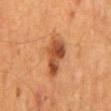• notes: catalogued during a skin exam; not biopsied
• image: 15 mm crop, total-body photography
• body site: the mid back
• patient: male, in their mid- to late 50s